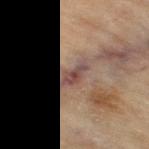No biopsy was performed on this lesion — it was imaged during a full skin examination and was not determined to be concerning. Cropped from a whole-body photographic skin survey; the tile spans about 15 mm. The subject is a female aged 78 to 82. The lesion is located on the right thigh.